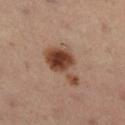| field | value |
|---|---|
| workup | catalogued during a skin exam; not biopsied |
| automated lesion analysis | a footprint of about 12 mm² and two-axis asymmetry of about 0.5; a mean CIELAB color near L≈42 a*≈20 b*≈28 and about 14 CIELAB-L* units darker than the surrounding skin; a classifier nevus-likeness of about 100/100 and a detector confidence of about 100 out of 100 that the crop contains a lesion |
| subject | male, roughly 55 years of age |
| imaging modality | ~15 mm crop, total-body skin-cancer survey |
| body site | the right lower leg |
| lighting | cross-polarized |
| lesion size | about 5.5 mm |